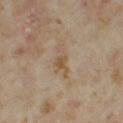This lesion was catalogued during total-body skin photography and was not selected for biopsy.
The patient is a female approximately 35 years of age.
Imaged with cross-polarized lighting.
Cropped from a total-body skin-imaging series; the visible field is about 15 mm.
An algorithmic analysis of the crop reported a mean CIELAB color near L≈50 a*≈13 b*≈29, a lesion–skin lightness drop of about 7, and a normalized border contrast of about 6. And it measured a border-irregularity rating of about 5.5/10, a color-variation rating of about 3.5/10, and radial color variation of about 1. And it measured a nevus-likeness score of about 0/100 and lesion-presence confidence of about 100/100.
From the right thigh.
Approximately 4 mm at its widest.